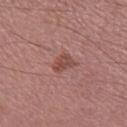| feature | finding |
|---|---|
| lesion diameter | about 3 mm |
| illumination | white-light |
| acquisition | total-body-photography crop, ~15 mm field of view |
| location | the right lower leg |
| subject | male, aged around 55 |
| automated lesion analysis | a mean CIELAB color near L≈47 a*≈24 b*≈25 and a normalized lesion–skin contrast near 6.5; a border-irregularity rating of about 3.5/10, internal color variation of about 2 on a 0–10 scale, and a peripheral color-asymmetry measure near 0.5 |